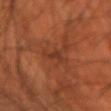Q: Was a biopsy performed?
A: no biopsy performed (imaged during a skin exam)
Q: What are the patient's age and sex?
A: male, roughly 70 years of age
Q: Where on the body is the lesion?
A: the left forearm
Q: How was the tile lit?
A: cross-polarized
Q: How large is the lesion?
A: ~3 mm (longest diameter)
Q: What is the imaging modality?
A: ~15 mm crop, total-body skin-cancer survey